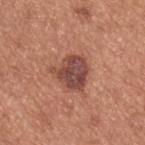Findings:
– workup — no biopsy performed (imaged during a skin exam)
– lighting — white-light illumination
– patient — male, in their mid- to late 50s
– diameter — ≈4 mm
– acquisition — ~15 mm tile from a whole-body skin photo
– site — the upper back